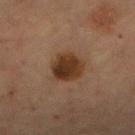patient: female, aged 58–62
image: total-body-photography crop, ~15 mm field of view
size: ≈3.5 mm
automated metrics: an area of roughly 9.5 mm², an outline eccentricity of about 0.5 (0 = round, 1 = elongated), and a symmetry-axis asymmetry near 0.15; a lesion–skin lightness drop of about 12 and a normalized lesion–skin contrast near 11.5
tile lighting: cross-polarized illumination
anatomic site: the abdomen